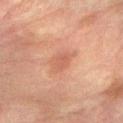Clinical impression:
Recorded during total-body skin imaging; not selected for excision or biopsy.
Context:
From the right forearm. Captured under cross-polarized illumination. About 3.5 mm across. Cropped from a total-body skin-imaging series; the visible field is about 15 mm. The subject is a male aged around 75. Automated tile analysis of the lesion measured a mean CIELAB color near L≈49 a*≈21 b*≈28, about 6 CIELAB-L* units darker than the surrounding skin, and a normalized border contrast of about 5. The software also gave a border-irregularity index near 3/10, internal color variation of about 2 on a 0–10 scale, and radial color variation of about 0.5. It also reported a nevus-likeness score of about 10/100 and a detector confidence of about 100 out of 100 that the crop contains a lesion.